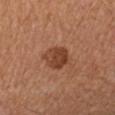Recorded during total-body skin imaging; not selected for excision or biopsy. A 15 mm close-up extracted from a 3D total-body photography capture. The recorded lesion diameter is about 3.5 mm. A female subject aged 33 to 37. From the right forearm. Imaged with cross-polarized lighting.